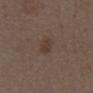The lesion was photographed on a routine skin check and not biopsied; there is no pathology result. Cropped from a whole-body photographic skin survey; the tile spans about 15 mm. The tile uses white-light illumination. A male patient, roughly 70 years of age. From the chest. An algorithmic analysis of the crop reported a footprint of about 3.5 mm², a shape eccentricity near 0.8, and a shape-asymmetry score of about 0.25 (0 = symmetric). The software also gave a border-irregularity index near 2.5/10, a color-variation rating of about 1.5/10, and radial color variation of about 0.5.